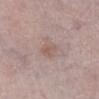Q: Is there a histopathology result?
A: imaged on a skin check; not biopsied
Q: What did automated image analysis measure?
A: a lesion area of about 5.5 mm², an eccentricity of roughly 0.7, and a symmetry-axis asymmetry near 0.15; an average lesion color of about L≈57 a*≈16 b*≈22 (CIELAB), about 6 CIELAB-L* units darker than the surrounding skin, and a normalized border contrast of about 5; a nevus-likeness score of about 0/100
Q: What is the lesion's diameter?
A: about 3 mm
Q: How was this image acquired?
A: ~15 mm tile from a whole-body skin photo
Q: How was the tile lit?
A: white-light
Q: Who is the patient?
A: female, about 65 years old
Q: Lesion location?
A: the right lower leg